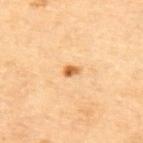Impression:
Recorded during total-body skin imaging; not selected for excision or biopsy.
Background:
A region of skin cropped from a whole-body photographic capture, roughly 15 mm wide. A female subject, aged 63–67. About 2 mm across. The total-body-photography lesion software estimated a footprint of about 2 mm² and a symmetry-axis asymmetry near 0.3. It also reported an average lesion color of about L≈65 a*≈25 b*≈46 (CIELAB). Located on the upper back. Imaged with cross-polarized lighting.